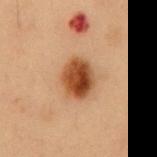follow-up = no biopsy performed (imaged during a skin exam); automated lesion analysis = a border-irregularity rating of about 1.5/10 and internal color variation of about 7 on a 0–10 scale; tile lighting = cross-polarized illumination; image source = total-body-photography crop, ~15 mm field of view; lesion size = ≈4 mm; location = the abdomen; patient = male, approximately 55 years of age.